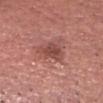{
  "biopsy_status": "not biopsied; imaged during a skin examination",
  "site": "head or neck",
  "lighting": "white-light",
  "patient": {
    "sex": "male",
    "age_approx": 55
  },
  "lesion_size": {
    "long_diameter_mm_approx": 3.5
  },
  "image": {
    "source": "total-body photography crop",
    "field_of_view_mm": 15
  }
}